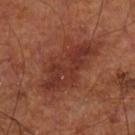Automated tile analysis of the lesion measured a mean CIELAB color near L≈33 a*≈25 b*≈27, a lesion–skin lightness drop of about 8, and a normalized border contrast of about 7. And it measured a border-irregularity index near 4/10, internal color variation of about 3 on a 0–10 scale, and a peripheral color-asymmetry measure near 1.
A region of skin cropped from a whole-body photographic capture, roughly 15 mm wide.
A male patient, about 70 years old.
The recorded lesion diameter is about 7.5 mm.
The tile uses cross-polarized illumination.
From the left lower leg.